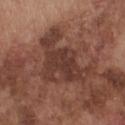Acquisition and patient details:
The recorded lesion diameter is about 8 mm. On the chest. This image is a 15 mm lesion crop taken from a total-body photograph. A male subject, aged 73 to 77.